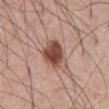  biopsy_status: not biopsied; imaged during a skin examination
  site: abdomen
  automated_metrics:
    cielab_L: 49
    cielab_a: 22
    cielab_b: 26
    vs_skin_darker_L: 15.0
    vs_skin_contrast_norm: 10.5
    color_variation_0_10: 6.0
    peripheral_color_asymmetry: 1.5
  lesion_size:
    long_diameter_mm_approx: 4.0
  image:
    source: total-body photography crop
    field_of_view_mm: 15
  lighting: white-light
  patient:
    sex: male
    age_approx: 60